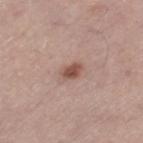Q: Is there a histopathology result?
A: imaged on a skin check; not biopsied